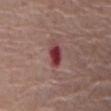Assessment: This lesion was catalogued during total-body skin photography and was not selected for biopsy. Image and clinical context: Located on the abdomen. This is a white-light tile. Longest diameter approximately 3 mm. Cropped from a whole-body photographic skin survey; the tile spans about 15 mm. An algorithmic analysis of the crop reported an area of roughly 5 mm², an outline eccentricity of about 0.8 (0 = round, 1 = elongated), and a shape-asymmetry score of about 0.2 (0 = symmetric). The analysis additionally found a lesion color around L≈39 a*≈30 b*≈19 in CIELAB, a lesion–skin lightness drop of about 14, and a normalized border contrast of about 11. A male subject, aged around 80.